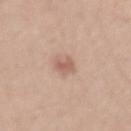Part of a total-body skin-imaging series; this lesion was reviewed on a skin check and was not flagged for biopsy. The recorded lesion diameter is about 2.5 mm. A 15 mm crop from a total-body photograph taken for skin-cancer surveillance. The lesion is on the arm. A male subject in their 40s. The total-body-photography lesion software estimated a lesion area of about 3.5 mm² and two-axis asymmetry of about 0.2. The software also gave a border-irregularity index near 2.5/10, internal color variation of about 1 on a 0–10 scale, and radial color variation of about 0.5. The analysis additionally found a nevus-likeness score of about 55/100 and lesion-presence confidence of about 100/100.